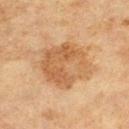<tbp_lesion>
  <biopsy_status>not biopsied; imaged during a skin examination</biopsy_status>
  <automated_metrics>
    <area_mm2_approx>28.0</area_mm2_approx>
    <shape_asymmetry>0.15</shape_asymmetry>
    <color_variation_0_10>5.0</color_variation_0_10>
    <peripheral_color_asymmetry>2.0</peripheral_color_asymmetry>
    <nevus_likeness_0_100>45</nevus_likeness_0_100>
  </automated_metrics>
  <image>
    <source>total-body photography crop</source>
    <field_of_view_mm>15</field_of_view_mm>
  </image>
  <lighting>cross-polarized</lighting>
  <site>left thigh</site>
  <lesion_size>
    <long_diameter_mm_approx>6.5</long_diameter_mm_approx>
  </lesion_size>
  <patient>
    <sex>female</sex>
    <age_approx>40</age_approx>
  </patient>
</tbp_lesion>